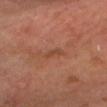{"patient": {"sex": "male", "age_approx": 60}, "image": {"source": "total-body photography crop", "field_of_view_mm": 15}, "site": "head or neck", "lesion_size": {"long_diameter_mm_approx": 2.5}, "automated_metrics": {"area_mm2_approx": 2.5, "eccentricity": 0.9, "nevus_likeness_0_100": 0, "lesion_detection_confidence_0_100": 95}}